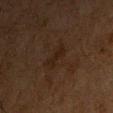Findings:
– workup — no biopsy performed (imaged during a skin exam)
– imaging modality — ~15 mm crop, total-body skin-cancer survey
– diameter — about 3 mm
– lighting — cross-polarized
– subject — male, aged around 75
– location — the chest